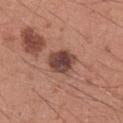Q: Was this lesion biopsied?
A: catalogued during a skin exam; not biopsied
Q: Illumination type?
A: white-light
Q: What are the patient's age and sex?
A: male, aged 33 to 37
Q: What is the lesion's diameter?
A: ~4 mm (longest diameter)
Q: Lesion location?
A: the right upper arm
Q: Automated lesion metrics?
A: a lesion color around L≈44 a*≈21 b*≈24 in CIELAB, a lesion–skin lightness drop of about 14, and a normalized lesion–skin contrast near 10.5; a border-irregularity rating of about 2/10 and a color-variation rating of about 5/10; a classifier nevus-likeness of about 0/100
Q: How was this image acquired?
A: total-body-photography crop, ~15 mm field of view